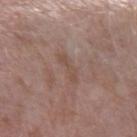Context:
Located on the left forearm. A close-up tile cropped from a whole-body skin photograph, about 15 mm across. Imaged with white-light lighting. A male patient, aged approximately 40.